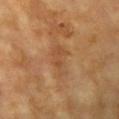Q: Is there a histopathology result?
A: catalogued during a skin exam; not biopsied
Q: What are the patient's age and sex?
A: female, approximately 75 years of age
Q: Lesion location?
A: the right upper arm
Q: Illumination type?
A: cross-polarized illumination
Q: Lesion size?
A: ≈3.5 mm
Q: How was this image acquired?
A: 15 mm crop, total-body photography